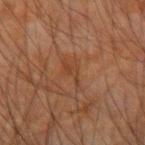This lesion was catalogued during total-body skin photography and was not selected for biopsy.
Captured under cross-polarized illumination.
A male patient, roughly 65 years of age.
From the right forearm.
About 3 mm across.
Automated tile analysis of the lesion measured a lesion area of about 3 mm², an eccentricity of roughly 0.9, and two-axis asymmetry of about 0.55. It also reported a peripheral color-asymmetry measure near 0. The software also gave a classifier nevus-likeness of about 0/100.
This image is a 15 mm lesion crop taken from a total-body photograph.